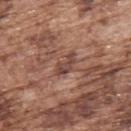Clinical impression: Imaged during a routine full-body skin examination; the lesion was not biopsied and no histopathology is available. Background: The lesion is located on the upper back. The subject is a male aged 73–77. A region of skin cropped from a whole-body photographic capture, roughly 15 mm wide.